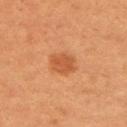{
  "biopsy_status": "not biopsied; imaged during a skin examination",
  "site": "left upper arm",
  "patient": {
    "sex": "female",
    "age_approx": 40
  },
  "image": {
    "source": "total-body photography crop",
    "field_of_view_mm": 15
  },
  "lesion_size": {
    "long_diameter_mm_approx": 3.0
  },
  "lighting": "cross-polarized"
}